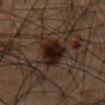{"biopsy_status": "not biopsied; imaged during a skin examination", "lighting": "cross-polarized", "image": {"source": "total-body photography crop", "field_of_view_mm": 15}, "automated_metrics": {"color_variation_0_10": 4.5, "nevus_likeness_0_100": 95, "lesion_detection_confidence_0_100": 95}, "patient": {"sex": "male", "age_approx": 65}, "lesion_size": {"long_diameter_mm_approx": 4.5}, "site": "chest"}